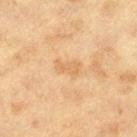{
  "biopsy_status": "not biopsied; imaged during a skin examination",
  "lighting": "cross-polarized",
  "site": "leg",
  "lesion_size": {
    "long_diameter_mm_approx": 3.0
  },
  "image": {
    "source": "total-body photography crop",
    "field_of_view_mm": 15
  },
  "patient": {
    "sex": "female",
    "age_approx": 40
  }
}